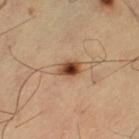Captured during whole-body skin photography for melanoma surveillance; the lesion was not biopsied.
An algorithmic analysis of the crop reported an area of roughly 4.5 mm², a shape eccentricity near 0.65, and a shape-asymmetry score of about 0.2 (0 = symmetric). The software also gave an average lesion color of about L≈46 a*≈21 b*≈33 (CIELAB), roughly 16 lightness units darker than nearby skin, and a lesion-to-skin contrast of about 11.5 (normalized; higher = more distinct). It also reported a border-irregularity rating of about 2/10, a color-variation rating of about 6.5/10, and peripheral color asymmetry of about 1.5. And it measured a nevus-likeness score of about 100/100 and lesion-presence confidence of about 100/100.
The lesion is on the left thigh.
A male subject aged around 60.
A close-up tile cropped from a whole-body skin photograph, about 15 mm across.
The tile uses cross-polarized illumination.
Longest diameter approximately 2.5 mm.